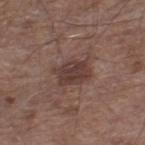Clinical impression:
Imaged during a routine full-body skin examination; the lesion was not biopsied and no histopathology is available.